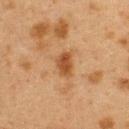Recorded during total-body skin imaging; not selected for excision or biopsy. The subject is a female approximately 40 years of age. The lesion is located on the upper back. A roughly 15 mm field-of-view crop from a total-body skin photograph. The lesion's longest dimension is about 3 mm. Captured under cross-polarized illumination.